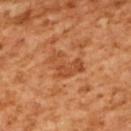Background:
A female patient, approximately 55 years of age. The lesion is located on the upper back. A 15 mm close-up extracted from a 3D total-body photography capture. This is a cross-polarized tile.